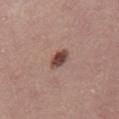{
  "biopsy_status": "not biopsied; imaged during a skin examination",
  "image": {
    "source": "total-body photography crop",
    "field_of_view_mm": 15
  },
  "lesion_size": {
    "long_diameter_mm_approx": 3.0
  },
  "site": "chest",
  "patient": {
    "sex": "male",
    "age_approx": 40
  },
  "automated_metrics": {
    "cielab_L": 45,
    "cielab_a": 21,
    "cielab_b": 23,
    "vs_skin_darker_L": 15.0,
    "vs_skin_contrast_norm": 11.0,
    "color_variation_0_10": 3.5,
    "peripheral_color_asymmetry": 1.0
  },
  "lighting": "white-light"
}